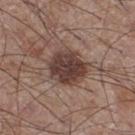Clinical impression: No biopsy was performed on this lesion — it was imaged during a full skin examination and was not determined to be concerning. Context: Located on the right thigh. Longest diameter approximately 5 mm. A male subject aged around 60. A region of skin cropped from a whole-body photographic capture, roughly 15 mm wide. This is a white-light tile.